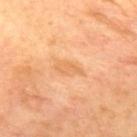workup: catalogued during a skin exam; not biopsied
imaging modality: 15 mm crop, total-body photography
anatomic site: the upper back
TBP lesion metrics: an area of roughly 4 mm² and two-axis asymmetry of about 0.35; an average lesion color of about L≈68 a*≈24 b*≈43 (CIELAB) and about 7 CIELAB-L* units darker than the surrounding skin; peripheral color asymmetry of about 0.5
lesion size: ~3.5 mm (longest diameter)
lighting: cross-polarized illumination
patient: female, aged 43 to 47